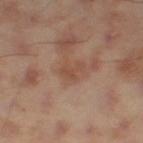| feature | finding |
|---|---|
| follow-up | catalogued during a skin exam; not biopsied |
| patient | male, aged around 45 |
| diameter | ≈3 mm |
| image source | ~15 mm tile from a whole-body skin photo |
| body site | the right thigh |
| illumination | cross-polarized |